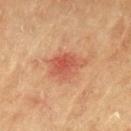Impression: The lesion was photographed on a routine skin check and not biopsied; there is no pathology result. Context: The patient is a female aged 78 to 82. Imaged with cross-polarized lighting. From the left upper arm. Longest diameter approximately 4 mm. The lesion-visualizer software estimated a footprint of about 8.5 mm² and a symmetry-axis asymmetry near 0.35. It also reported a within-lesion color-variation index near 4/10 and peripheral color asymmetry of about 1.5. The analysis additionally found an automated nevus-likeness rating near 40 out of 100 and lesion-presence confidence of about 100/100. This image is a 15 mm lesion crop taken from a total-body photograph.